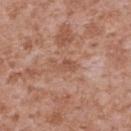Q: Was this lesion biopsied?
A: imaged on a skin check; not biopsied
Q: Patient demographics?
A: male, approximately 45 years of age
Q: What is the imaging modality?
A: total-body-photography crop, ~15 mm field of view
Q: Automated lesion metrics?
A: a lesion area of about 3.5 mm² and an outline eccentricity of about 0.9 (0 = round, 1 = elongated); an average lesion color of about L≈53 a*≈23 b*≈30 (CIELAB) and a lesion–skin lightness drop of about 7; a border-irregularity rating of about 5/10 and radial color variation of about 0.5; a nevus-likeness score of about 0/100 and lesion-presence confidence of about 95/100
Q: How was the tile lit?
A: white-light illumination
Q: Lesion size?
A: ~3 mm (longest diameter)
Q: Lesion location?
A: the back